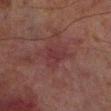{
  "biopsy_status": "not biopsied; imaged during a skin examination",
  "image": {
    "source": "total-body photography crop",
    "field_of_view_mm": 15
  },
  "site": "leg",
  "patient": {
    "sex": "male",
    "age_approx": 70
  },
  "lighting": "cross-polarized"
}